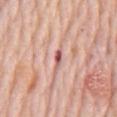Clinical impression:
Recorded during total-body skin imaging; not selected for excision or biopsy.
Image and clinical context:
A roughly 15 mm field-of-view crop from a total-body skin photograph. The lesion-visualizer software estimated a footprint of about 2 mm², a shape eccentricity near 0.95, and two-axis asymmetry of about 0.35. And it measured a border-irregularity rating of about 4/10, a color-variation rating of about 0/10, and peripheral color asymmetry of about 0. The analysis additionally found an automated nevus-likeness rating near 0 out of 100 and a detector confidence of about 65 out of 100 that the crop contains a lesion. The lesion is located on the chest. A male patient, about 80 years old. Measured at roughly 2.5 mm in maximum diameter. Imaged with white-light lighting.